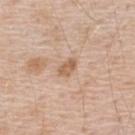Case summary:
* subject · male, aged around 50
* imaging modality · ~15 mm crop, total-body skin-cancer survey
* tile lighting · white-light
* site · the upper back
* size · ≈2.5 mm